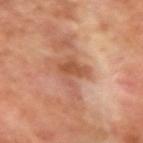Imaged during a routine full-body skin examination; the lesion was not biopsied and no histopathology is available. The patient is a male aged 68 to 72. About 4.5 mm across. A lesion tile, about 15 mm wide, cut from a 3D total-body photograph. Located on the right upper arm. Captured under cross-polarized illumination.